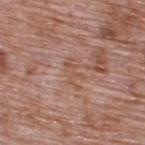A region of skin cropped from a whole-body photographic capture, roughly 15 mm wide. Captured under white-light illumination. The lesion is located on the upper back. A male subject aged around 70.